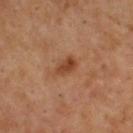Imaged during a routine full-body skin examination; the lesion was not biopsied and no histopathology is available. The subject is a male in their mid-50s. Captured under cross-polarized illumination. Approximately 3 mm at its widest. A 15 mm close-up tile from a total-body photography series done for melanoma screening. The lesion is on the back.